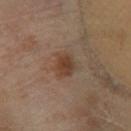follow-up = catalogued during a skin exam; not biopsied.